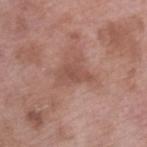| feature | finding |
|---|---|
| biopsy status | total-body-photography surveillance lesion; no biopsy |
| automated lesion analysis | a lesion area of about 6.5 mm², an eccentricity of roughly 0.7, and two-axis asymmetry of about 0.5; about 8 CIELAB-L* units darker than the surrounding skin and a normalized lesion–skin contrast near 5.5 |
| site | the right lower leg |
| acquisition | 15 mm crop, total-body photography |
| lighting | white-light |
| lesion size | ~4 mm (longest diameter) |
| subject | female, aged 68 to 72 |